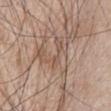Part of a total-body skin-imaging series; this lesion was reviewed on a skin check and was not flagged for biopsy.
The lesion is located on the chest.
Imaged with white-light lighting.
Measured at roughly 5 mm in maximum diameter.
The lesion-visualizer software estimated a lesion–skin lightness drop of about 7 and a normalized border contrast of about 4.5. It also reported border irregularity of about 9.5 on a 0–10 scale, a within-lesion color-variation index near 4.5/10, and a peripheral color-asymmetry measure near 1.5.
A 15 mm close-up tile from a total-body photography series done for melanoma screening.
A male subject, in their mid-60s.